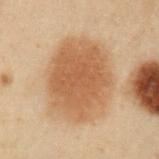Impression:
The lesion was tiled from a total-body skin photograph and was not biopsied.
Clinical summary:
A 15 mm close-up tile from a total-body photography series done for melanoma screening. This is a cross-polarized tile. The lesion is on the left upper arm. The patient is a male roughly 55 years of age. The total-body-photography lesion software estimated a mean CIELAB color near L≈47 a*≈17 b*≈31, a lesion–skin lightness drop of about 10, and a normalized lesion–skin contrast near 8. The analysis additionally found border irregularity of about 2 on a 0–10 scale and radial color variation of about 0.5. The software also gave a nevus-likeness score of about 80/100. About 8 mm across.